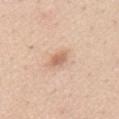Q: Was a biopsy performed?
A: imaged on a skin check; not biopsied
Q: Lesion location?
A: the chest
Q: What is the imaging modality?
A: total-body-photography crop, ~15 mm field of view
Q: Who is the patient?
A: female, roughly 40 years of age